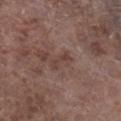Recorded during total-body skin imaging; not selected for excision or biopsy. On the right lower leg. A region of skin cropped from a whole-body photographic capture, roughly 15 mm wide. Captured under white-light illumination. A male patient, in their 70s.